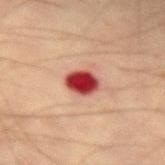Clinical impression: Captured during whole-body skin photography for melanoma surveillance; the lesion was not biopsied. Background: The tile uses cross-polarized illumination. The lesion-visualizer software estimated a lesion color around L≈38 a*≈35 b*≈27 in CIELAB, roughly 21 lightness units darker than nearby skin, and a lesion-to-skin contrast of about 15.5 (normalized; higher = more distinct). About 3 mm across. Located on the right forearm. This image is a 15 mm lesion crop taken from a total-body photograph. A male patient in their mid-50s.